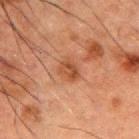Case summary:
- workup · no biopsy performed (imaged during a skin exam)
- subject · male, approximately 50 years of age
- TBP lesion metrics · an area of roughly 4.5 mm², an eccentricity of roughly 0.65, and a shape-asymmetry score of about 0.3 (0 = symmetric); a mean CIELAB color near L≈39 a*≈21 b*≈30, roughly 8 lightness units darker than nearby skin, and a lesion-to-skin contrast of about 7.5 (normalized; higher = more distinct); a color-variation rating of about 3.5/10 and a peripheral color-asymmetry measure near 1.5; a nevus-likeness score of about 20/100 and a lesion-detection confidence of about 100/100
- size · about 2.5 mm
- location · the back
- acquisition · ~15 mm crop, total-body skin-cancer survey